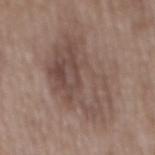{
  "biopsy_status": "not biopsied; imaged during a skin examination",
  "patient": {
    "sex": "male",
    "age_approx": 50
  },
  "image": {
    "source": "total-body photography crop",
    "field_of_view_mm": 15
  },
  "site": "mid back"
}